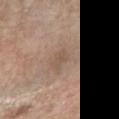biopsy status = catalogued during a skin exam; not biopsied | anatomic site = the right forearm | acquisition = total-body-photography crop, ~15 mm field of view | image-analysis metrics = a mean CIELAB color near L≈53 a*≈15 b*≈28, roughly 6 lightness units darker than nearby skin, and a normalized border contrast of about 5 | tile lighting = white-light illumination | subject = female, aged 53 to 57.